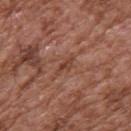Captured during whole-body skin photography for melanoma surveillance; the lesion was not biopsied.
The tile uses white-light illumination.
A 15 mm close-up extracted from a 3D total-body photography capture.
The total-body-photography lesion software estimated an area of roughly 2.5 mm², an outline eccentricity of about 0.95 (0 = round, 1 = elongated), and a shape-asymmetry score of about 0.35 (0 = symmetric). The analysis additionally found an average lesion color of about L≈42 a*≈23 b*≈29 (CIELAB), a lesion–skin lightness drop of about 8, and a lesion-to-skin contrast of about 6.5 (normalized; higher = more distinct).
From the upper back.
Approximately 3 mm at its widest.
A male subject, in their mid-70s.